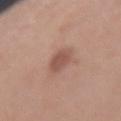<record>
<biopsy_status>not biopsied; imaged during a skin examination</biopsy_status>
</record>